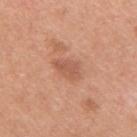This is a white-light tile. The lesion's longest dimension is about 4 mm. A roughly 15 mm field-of-view crop from a total-body skin photograph. The subject is a male approximately 30 years of age. The lesion is on the right upper arm. The total-body-photography lesion software estimated an outline eccentricity of about 0.85 (0 = round, 1 = elongated) and a shape-asymmetry score of about 0.25 (0 = symmetric). The software also gave a lesion color around L≈57 a*≈24 b*≈32 in CIELAB and about 9 CIELAB-L* units darker than the surrounding skin.